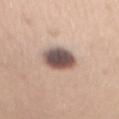biopsy status = imaged on a skin check; not biopsied
size = ≈4 mm
image source = total-body-photography crop, ~15 mm field of view
body site = the chest
subject = female, in their 30s
tile lighting = white-light illumination
automated lesion analysis = a within-lesion color-variation index near 7/10 and radial color variation of about 2